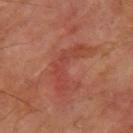No biopsy was performed on this lesion — it was imaged during a full skin examination and was not determined to be concerning. A male patient, aged around 70. On the right thigh. The lesion's longest dimension is about 6.5 mm. A 15 mm close-up tile from a total-body photography series done for melanoma screening.